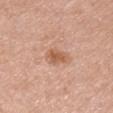Part of a total-body skin-imaging series; this lesion was reviewed on a skin check and was not flagged for biopsy.
Automated tile analysis of the lesion measured a lesion-detection confidence of about 100/100.
A female patient, aged 48 to 52.
The lesion is located on the chest.
Captured under white-light illumination.
Cropped from a whole-body photographic skin survey; the tile spans about 15 mm.